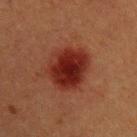follow-up — no biopsy performed (imaged during a skin exam)
TBP lesion metrics — an area of roughly 18 mm², an eccentricity of roughly 0.55, and a shape-asymmetry score of about 0.15 (0 = symmetric); an average lesion color of about L≈23 a*≈26 b*≈25 (CIELAB), about 11 CIELAB-L* units darker than the surrounding skin, and a lesion-to-skin contrast of about 11.5 (normalized; higher = more distinct); border irregularity of about 1.5 on a 0–10 scale and a peripheral color-asymmetry measure near 1; a detector confidence of about 100 out of 100 that the crop contains a lesion
location — the upper back
imaging modality — 15 mm crop, total-body photography
lighting — cross-polarized
patient — male, aged 38 to 42